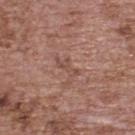No biopsy was performed on this lesion — it was imaged during a full skin examination and was not determined to be concerning.
This is a white-light tile.
The patient is a female about 65 years old.
Cropped from a total-body skin-imaging series; the visible field is about 15 mm.
On the back.
Longest diameter approximately 3 mm.